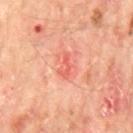Q: What is the anatomic site?
A: the mid back
Q: What is the imaging modality?
A: total-body-photography crop, ~15 mm field of view
Q: What are the patient's age and sex?
A: male, aged approximately 65